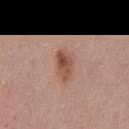– biopsy status: imaged on a skin check; not biopsied
– tile lighting: white-light
– lesion size: about 4 mm
– acquisition: total-body-photography crop, ~15 mm field of view
– patient: male, about 55 years old
– anatomic site: the mid back